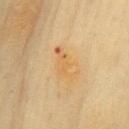Findings:
* diameter: ≈4.5 mm
* image source: 15 mm crop, total-body photography
* location: the front of the torso
* patient: female, in their 60s